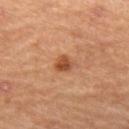notes: imaged on a skin check; not biopsied | image source: 15 mm crop, total-body photography | diameter: ~2.5 mm (longest diameter) | lighting: cross-polarized illumination | location: the left thigh | automated metrics: an area of roughly 4 mm² and a shape eccentricity near 0.55; a lesion–skin lightness drop of about 11 and a normalized lesion–skin contrast near 8; a border-irregularity rating of about 1.5/10 and a within-lesion color-variation index near 3/10 | subject: male, aged approximately 60.